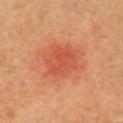| feature | finding |
|---|---|
| biopsy status | no biopsy performed (imaged during a skin exam) |
| automated lesion analysis | a lesion area of about 15 mm² and two-axis asymmetry of about 0.2; a mean CIELAB color near L≈57 a*≈36 b*≈39 and a normalized border contrast of about 5; a border-irregularity rating of about 3/10, internal color variation of about 3 on a 0–10 scale, and peripheral color asymmetry of about 1 |
| lesion diameter | ≈4.5 mm |
| lighting | cross-polarized illumination |
| patient | female, aged 68 to 72 |
| location | the right upper arm |
| acquisition | total-body-photography crop, ~15 mm field of view |